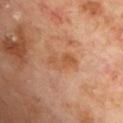Q: Was a biopsy performed?
A: catalogued during a skin exam; not biopsied
Q: What lighting was used for the tile?
A: cross-polarized
Q: What is the imaging modality?
A: ~15 mm crop, total-body skin-cancer survey
Q: What did automated image analysis measure?
A: a lesion color around L≈53 a*≈23 b*≈36 in CIELAB, about 7 CIELAB-L* units darker than the surrounding skin, and a normalized border contrast of about 6; a border-irregularity rating of about 3.5/10, a color-variation rating of about 4/10, and peripheral color asymmetry of about 1; a nevus-likeness score of about 0/100 and a lesion-detection confidence of about 100/100
Q: Lesion size?
A: ~3.5 mm (longest diameter)
Q: What is the anatomic site?
A: the chest
Q: What are the patient's age and sex?
A: male, about 70 years old